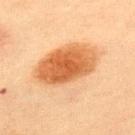Clinical impression: No biopsy was performed on this lesion — it was imaged during a full skin examination and was not determined to be concerning. Image and clinical context: A region of skin cropped from a whole-body photographic capture, roughly 15 mm wide. The subject is a male approximately 60 years of age. Located on the upper back. This is a cross-polarized tile. Approximately 8.5 mm at its widest.